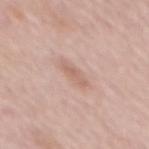Clinical impression:
The lesion was photographed on a routine skin check and not biopsied; there is no pathology result.
Background:
The recorded lesion diameter is about 3.5 mm. A 15 mm crop from a total-body photograph taken for skin-cancer surveillance. A female patient approximately 65 years of age. On the mid back. Captured under white-light illumination.